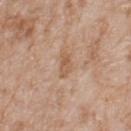Clinical impression: The lesion was photographed on a routine skin check and not biopsied; there is no pathology result. Acquisition and patient details: On the upper back. Longest diameter approximately 3 mm. Captured under white-light illumination. A roughly 15 mm field-of-view crop from a total-body skin photograph. A male subject approximately 80 years of age.